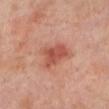Recorded during total-body skin imaging; not selected for excision or biopsy.
The lesion-visualizer software estimated an area of roughly 10 mm², an eccentricity of roughly 0.5, and two-axis asymmetry of about 0.3. The analysis additionally found a lesion color around L≈53 a*≈28 b*≈30 in CIELAB, about 11 CIELAB-L* units darker than the surrounding skin, and a lesion-to-skin contrast of about 7.5 (normalized; higher = more distinct). And it measured a within-lesion color-variation index near 3.5/10.
A 15 mm close-up extracted from a 3D total-body photography capture.
From the leg.
A female subject about 50 years old.
The lesion's longest dimension is about 4 mm.